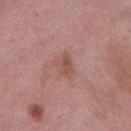  biopsy_status: not biopsied; imaged during a skin examination
  image:
    source: total-body photography crop
    field_of_view_mm: 15
  lighting: white-light
  patient:
    sex: female
    age_approx: 50
  lesion_size:
    long_diameter_mm_approx: 3.0
  site: left thigh